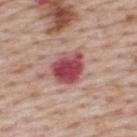• notes — catalogued during a skin exam; not biopsied
• body site — the upper back
• size — ~4.5 mm (longest diameter)
• subject — male, aged around 65
• acquisition — total-body-photography crop, ~15 mm field of view